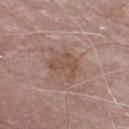Part of a total-body skin-imaging series; this lesion was reviewed on a skin check and was not flagged for biopsy. The lesion's longest dimension is about 3.5 mm. This is a white-light tile. Cropped from a total-body skin-imaging series; the visible field is about 15 mm. Automated image analysis of the tile measured border irregularity of about 6.5 on a 0–10 scale, a color-variation rating of about 2/10, and radial color variation of about 0.5. The lesion is on the chest. A male patient aged 73–77.